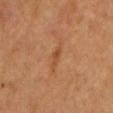Captured during whole-body skin photography for melanoma surveillance; the lesion was not biopsied.
A male subject, roughly 55 years of age.
From the front of the torso.
A 15 mm close-up extracted from a 3D total-body photography capture.
Imaged with cross-polarized lighting.